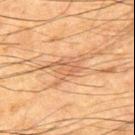Clinical impression:
This lesion was catalogued during total-body skin photography and was not selected for biopsy.
Background:
The patient is a male approximately 65 years of age. A region of skin cropped from a whole-body photographic capture, roughly 15 mm wide. From the upper back. Captured under cross-polarized illumination.